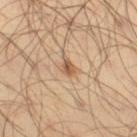<tbp_lesion>
  <biopsy_status>not biopsied; imaged during a skin examination</biopsy_status>
  <lesion_size>
    <long_diameter_mm_approx>2.0</long_diameter_mm_approx>
  </lesion_size>
  <site>right thigh</site>
  <lighting>cross-polarized</lighting>
  <patient>
    <sex>male</sex>
    <age_approx>45</age_approx>
  </patient>
  <automated_metrics>
    <area_mm2_approx>2.5</area_mm2_approx>
    <eccentricity>0.7</eccentricity>
    <border_irregularity_0_10>1.5</border_irregularity_0_10>
    <peripheral_color_asymmetry>1.0</peripheral_color_asymmetry>
  </automated_metrics>
  <image>
    <source>total-body photography crop</source>
    <field_of_view_mm>15</field_of_view_mm>
  </image>
</tbp_lesion>